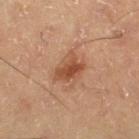<lesion>
  <biopsy_status>not biopsied; imaged during a skin examination</biopsy_status>
  <image>
    <source>total-body photography crop</source>
    <field_of_view_mm>15</field_of_view_mm>
  </image>
  <patient>
    <sex>male</sex>
    <age_approx>60</age_approx>
  </patient>
  <site>left thigh</site>
  <lesion_size>
    <long_diameter_mm_approx>3.5</long_diameter_mm_approx>
  </lesion_size>
</lesion>